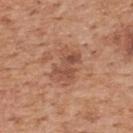Captured during whole-body skin photography for melanoma surveillance; the lesion was not biopsied.
A male subject aged 58–62.
Longest diameter approximately 4 mm.
Captured under white-light illumination.
A roughly 15 mm field-of-view crop from a total-body skin photograph.
The lesion is on the upper back.